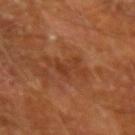Clinical impression: Recorded during total-body skin imaging; not selected for excision or biopsy. Context: The lesion is on the left forearm. The tile uses cross-polarized illumination. Cropped from a total-body skin-imaging series; the visible field is about 15 mm. The lesion's longest dimension is about 4 mm. A male subject about 65 years old.